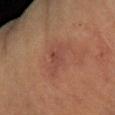Notes:
– notes · total-body-photography surveillance lesion; no biopsy
– patient · female, in their 60s
– acquisition · total-body-photography crop, ~15 mm field of view
– tile lighting · cross-polarized illumination
– lesion diameter · about 4 mm
– TBP lesion metrics · a mean CIELAB color near L≈40 a*≈21 b*≈24, about 5 CIELAB-L* units darker than the surrounding skin, and a lesion-to-skin contrast of about 5 (normalized; higher = more distinct); a border-irregularity rating of about 5/10, a within-lesion color-variation index near 2/10, and peripheral color asymmetry of about 0.5
– anatomic site · the right forearm